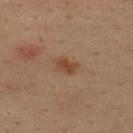Assessment:
Part of a total-body skin-imaging series; this lesion was reviewed on a skin check and was not flagged for biopsy.
Background:
The subject is a male aged around 35. Located on the back. A 15 mm close-up extracted from a 3D total-body photography capture.